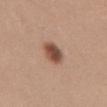The lesion was photographed on a routine skin check and not biopsied; there is no pathology result. Measured at roughly 3 mm in maximum diameter. This is a white-light tile. A male subject roughly 30 years of age. Automated image analysis of the tile measured roughly 15 lightness units darker than nearby skin and a lesion-to-skin contrast of about 10.5 (normalized; higher = more distinct). The software also gave a nevus-likeness score of about 100/100. A 15 mm close-up tile from a total-body photography series done for melanoma screening. The lesion is located on the abdomen.